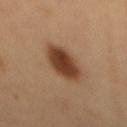This lesion was catalogued during total-body skin photography and was not selected for biopsy.
The patient is a male roughly 50 years of age.
Located on the back.
Cropped from a whole-body photographic skin survey; the tile spans about 15 mm.
Captured under cross-polarized illumination.